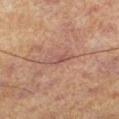Clinical impression:
Captured during whole-body skin photography for melanoma surveillance; the lesion was not biopsied.
Image and clinical context:
Cropped from a total-body skin-imaging series; the visible field is about 15 mm. The lesion is on the left lower leg. A male subject in their mid-60s.